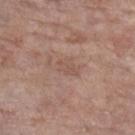body site=the left lower leg
image=~15 mm crop, total-body skin-cancer survey
subject=female, roughly 85 years of age or older